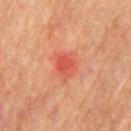Notes:
* workup — no biopsy performed (imaged during a skin exam)
* image — ~15 mm crop, total-body skin-cancer survey
* body site — the chest
* patient — male, aged 73–77
* tile lighting — cross-polarized illumination
* automated metrics — a footprint of about 6 mm², an outline eccentricity of about 0.7 (0 = round, 1 = elongated), and a symmetry-axis asymmetry near 0.2; a lesion-detection confidence of about 100/100